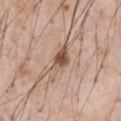<lesion>
<lighting>white-light</lighting>
<site>abdomen</site>
<image>
  <source>total-body photography crop</source>
  <field_of_view_mm>15</field_of_view_mm>
</image>
<automated_metrics>
  <border_irregularity_0_10>4.0</border_irregularity_0_10>
  <color_variation_0_10>7.0</color_variation_0_10>
  <peripheral_color_asymmetry>2.5</peripheral_color_asymmetry>
  <nevus_likeness_0_100>95</nevus_likeness_0_100>
  <lesion_detection_confidence_0_100>100</lesion_detection_confidence_0_100>
</automated_metrics>
<patient>
  <sex>male</sex>
  <age_approx>55</age_approx>
</patient>
<lesion_size>
  <long_diameter_mm_approx>5.0</long_diameter_mm_approx>
</lesion_size>
</lesion>